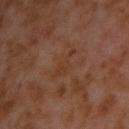<tbp_lesion>
<biopsy_status>not biopsied; imaged during a skin examination</biopsy_status>
<lesion_size>
  <long_diameter_mm_approx>4.0</long_diameter_mm_approx>
</lesion_size>
<image>
  <source>total-body photography crop</source>
  <field_of_view_mm>15</field_of_view_mm>
</image>
<lighting>cross-polarized</lighting>
<site>upper back</site>
<patient>
  <sex>male</sex>
  <age_approx>60</age_approx>
</patient>
<automated_metrics>
  <area_mm2_approx>5.0</area_mm2_approx>
  <eccentricity>0.9</eccentricity>
  <shape_asymmetry>0.4</shape_asymmetry>
  <cielab_L>33</cielab_L>
  <cielab_a>19</cielab_a>
  <cielab_b>27</cielab_b>
  <vs_skin_darker_L>4.0</vs_skin_darker_L>
  <vs_skin_contrast_norm>4.5</vs_skin_contrast_norm>
  <nevus_likeness_0_100>0</nevus_likeness_0_100>
  <lesion_detection_confidence_0_100>100</lesion_detection_confidence_0_100>
</automated_metrics>
</tbp_lesion>